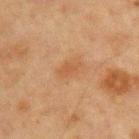Imaged during a routine full-body skin examination; the lesion was not biopsied and no histopathology is available. The lesion is located on the chest. Longest diameter approximately 3 mm. Automated tile analysis of the lesion measured a lesion color around L≈44 a*≈19 b*≈32 in CIELAB, a lesion–skin lightness drop of about 6, and a normalized lesion–skin contrast near 5.5. Imaged with cross-polarized lighting. The subject is a male aged approximately 65. Cropped from a whole-body photographic skin survey; the tile spans about 15 mm.